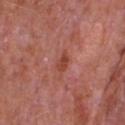Clinical impression:
Captured during whole-body skin photography for melanoma surveillance; the lesion was not biopsied.
Background:
The tile uses white-light illumination. A lesion tile, about 15 mm wide, cut from a 3D total-body photograph. The recorded lesion diameter is about 2.5 mm. A male subject aged 63–67. On the chest. The lesion-visualizer software estimated a footprint of about 3 mm² and a shape-asymmetry score of about 0.25 (0 = symmetric). The analysis additionally found a mean CIELAB color near L≈45 a*≈29 b*≈31, a lesion–skin lightness drop of about 8, and a lesion-to-skin contrast of about 7.5 (normalized; higher = more distinct). The software also gave a border-irregularity index near 2/10 and radial color variation of about 1.